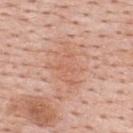| feature | finding |
|---|---|
| notes | imaged on a skin check; not biopsied |
| lesion diameter | ~5 mm (longest diameter) |
| image source | total-body-photography crop, ~15 mm field of view |
| image-analysis metrics | about 6 CIELAB-L* units darker than the surrounding skin and a lesion-to-skin contrast of about 4.5 (normalized; higher = more distinct); border irregularity of about 7 on a 0–10 scale, internal color variation of about 3 on a 0–10 scale, and radial color variation of about 1 |
| subject | male, aged 53–57 |
| site | the upper back |